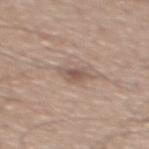  biopsy_status: not biopsied; imaged during a skin examination
  automated_metrics:
    eccentricity: 0.85
    shape_asymmetry: 0.35
  image:
    source: total-body photography crop
    field_of_view_mm: 15
  site: upper back
  patient:
    sex: male
    age_approx: 60